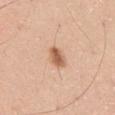Findings:
• biopsy status — total-body-photography surveillance lesion; no biopsy
• body site — the left thigh
• lighting — white-light illumination
• subject — male, about 50 years old
• acquisition — total-body-photography crop, ~15 mm field of view